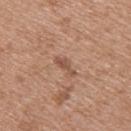Q: Lesion location?
A: the upper back
Q: Automated lesion metrics?
A: border irregularity of about 4.5 on a 0–10 scale, a within-lesion color-variation index near 1/10, and radial color variation of about 0.5
Q: How large is the lesion?
A: ~3 mm (longest diameter)
Q: Who is the patient?
A: female, aged around 45
Q: What kind of image is this?
A: ~15 mm tile from a whole-body skin photo
Q: How was the tile lit?
A: white-light